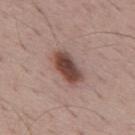{
  "image": {
    "source": "total-body photography crop",
    "field_of_view_mm": 15
  },
  "patient": {
    "sex": "male",
    "age_approx": 70
  },
  "site": "mid back"
}